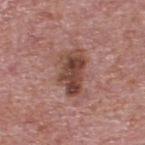Recorded during total-body skin imaging; not selected for excision or biopsy. Captured under white-light illumination. A 15 mm close-up extracted from a 3D total-body photography capture. A male patient aged 73 to 77. The lesion is located on the upper back. Automated tile analysis of the lesion measured an average lesion color of about L≈44 a*≈22 b*≈25 (CIELAB), roughly 13 lightness units darker than nearby skin, and a normalized border contrast of about 10. The recorded lesion diameter is about 5 mm.